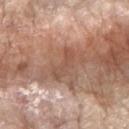biopsy_status: not biopsied; imaged during a skin examination
image:
  source: total-body photography crop
  field_of_view_mm: 15
patient:
  sex: female
  age_approx: 75
site: left forearm